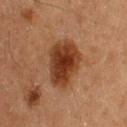Recorded during total-body skin imaging; not selected for excision or biopsy.
The lesion's longest dimension is about 5 mm.
A male subject approximately 60 years of age.
A roughly 15 mm field-of-view crop from a total-body skin photograph.
The tile uses cross-polarized illumination.